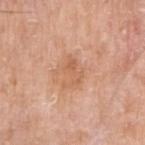biopsy status — no biopsy performed (imaged during a skin exam) | image source — total-body-photography crop, ~15 mm field of view | TBP lesion metrics — an outline eccentricity of about 0.75 (0 = round, 1 = elongated); border irregularity of about 7 on a 0–10 scale and a color-variation rating of about 3.5/10; an automated nevus-likeness rating near 0 out of 100 | lighting — white-light illumination | size — about 3.5 mm | location — the right upper arm | subject — male, in their mid- to late 60s.